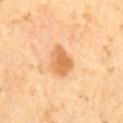{
  "biopsy_status": "not biopsied; imaged during a skin examination",
  "lighting": "cross-polarized",
  "lesion_size": {
    "long_diameter_mm_approx": 3.5
  },
  "automated_metrics": {
    "border_irregularity_0_10": 2.5,
    "color_variation_0_10": 3.0,
    "peripheral_color_asymmetry": 1.0
  },
  "image": {
    "source": "total-body photography crop",
    "field_of_view_mm": 15
  },
  "patient": {
    "sex": "male",
    "age_approx": 65
  }
}